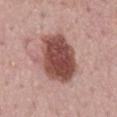<record>
  <biopsy_status>not biopsied; imaged during a skin examination</biopsy_status>
  <patient>
    <sex>male</sex>
    <age_approx>50</age_approx>
  </patient>
  <lighting>white-light</lighting>
  <image>
    <source>total-body photography crop</source>
    <field_of_view_mm>15</field_of_view_mm>
  </image>
  <lesion_size>
    <long_diameter_mm_approx>6.5</long_diameter_mm_approx>
  </lesion_size>
  <site>back</site>
</record>